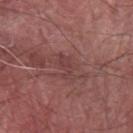Impression:
The lesion was tiled from a total-body skin photograph and was not biopsied.
Image and clinical context:
The lesion is located on the left forearm. This is a white-light tile. The patient is a male aged 58–62. The lesion-visualizer software estimated a border-irregularity rating of about 5/10, internal color variation of about 1.5 on a 0–10 scale, and a peripheral color-asymmetry measure near 0.5. A close-up tile cropped from a whole-body skin photograph, about 15 mm across.